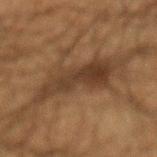<lesion>
<biopsy_status>not biopsied; imaged during a skin examination</biopsy_status>
</lesion>